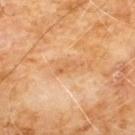{"biopsy_status": "not biopsied; imaged during a skin examination", "site": "chest", "image": {"source": "total-body photography crop", "field_of_view_mm": 15}, "automated_metrics": {"area_mm2_approx": 3.0, "eccentricity": 0.9, "shape_asymmetry": 0.25, "nevus_likeness_0_100": 0, "lesion_detection_confidence_0_100": 100}, "patient": {"sex": "male", "age_approx": 60}}